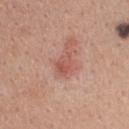Imaged during a routine full-body skin examination; the lesion was not biopsied and no histopathology is available. On the upper back. A female patient aged 38 to 42. Captured under white-light illumination. Cropped from a whole-body photographic skin survey; the tile spans about 15 mm. Automated tile analysis of the lesion measured a shape-asymmetry score of about 0.25 (0 = symmetric). The software also gave a lesion color around L≈55 a*≈26 b*≈28 in CIELAB, a lesion–skin lightness drop of about 8, and a normalized border contrast of about 6. The analysis additionally found a border-irregularity rating of about 2.5/10, a color-variation rating of about 3/10, and radial color variation of about 1.